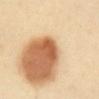TBP lesion metrics: a lesion area of about 3.5 mm², a shape eccentricity near 0.8, and a symmetry-axis asymmetry near 0.3; roughly 13 lightness units darker than nearby skin and a normalized lesion–skin contrast near 9; a classifier nevus-likeness of about 100/100 and lesion-presence confidence of about 100/100 | image: ~15 mm crop, total-body skin-cancer survey | body site: the abdomen | size: ≈2.5 mm | patient: female, approximately 40 years of age | illumination: cross-polarized illumination.